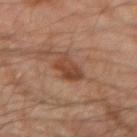follow-up: total-body-photography surveillance lesion; no biopsy | patient: male, aged around 45 | anatomic site: the left forearm | image-analysis metrics: a footprint of about 6.5 mm², an outline eccentricity of about 0.5 (0 = round, 1 = elongated), and two-axis asymmetry of about 0.2; a border-irregularity rating of about 2.5/10 and a peripheral color-asymmetry measure near 1 | size: ≈3 mm | imaging modality: 15 mm crop, total-body photography.